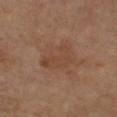Imaged during a routine full-body skin examination; the lesion was not biopsied and no histopathology is available.
Measured at roughly 4.5 mm in maximum diameter.
A close-up tile cropped from a whole-body skin photograph, about 15 mm across.
A male patient, aged around 70.
An algorithmic analysis of the crop reported an area of roughly 8 mm², a shape eccentricity near 0.8, and a symmetry-axis asymmetry near 0.55. The analysis additionally found a mean CIELAB color near L≈42 a*≈19 b*≈29 and a normalized border contrast of about 5. The analysis additionally found border irregularity of about 6.5 on a 0–10 scale, a within-lesion color-variation index near 2/10, and a peripheral color-asymmetry measure near 0.5.
From the arm.